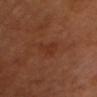This lesion was catalogued during total-body skin photography and was not selected for biopsy.
A region of skin cropped from a whole-body photographic capture, roughly 15 mm wide.
A male patient roughly 50 years of age.
The lesion is on the head or neck.